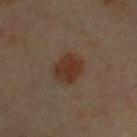lesion diameter=~3.5 mm (longest diameter); lighting=cross-polarized; location=the front of the torso; patient=male, aged 53–57; imaging modality=~15 mm crop, total-body skin-cancer survey.